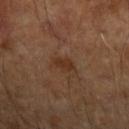{"biopsy_status": "not biopsied; imaged during a skin examination", "patient": {"sex": "male", "age_approx": 65}, "image": {"source": "total-body photography crop", "field_of_view_mm": 15}, "lighting": "cross-polarized", "lesion_size": {"long_diameter_mm_approx": 2.5}, "site": "left forearm", "automated_metrics": {"cielab_L": 31, "cielab_a": 18, "cielab_b": 27, "vs_skin_darker_L": 6.0, "vs_skin_contrast_norm": 7.0, "border_irregularity_0_10": 2.5, "color_variation_0_10": 1.0}}